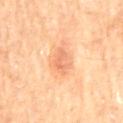{
  "biopsy_status": "not biopsied; imaged during a skin examination",
  "image": {
    "source": "total-body photography crop",
    "field_of_view_mm": 15
  },
  "site": "mid back",
  "patient": {
    "sex": "male",
    "age_approx": "85+"
  },
  "lesion_size": {
    "long_diameter_mm_approx": 3.5
  },
  "automated_metrics": {
    "shape_asymmetry": 0.4
  },
  "lighting": "cross-polarized"
}